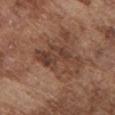Imaged during a routine full-body skin examination; the lesion was not biopsied and no histopathology is available. Located on the chest. Imaged with white-light lighting. A 15 mm close-up tile from a total-body photography series done for melanoma screening. The recorded lesion diameter is about 6 mm. Automated tile analysis of the lesion measured an area of roughly 12 mm², a shape eccentricity near 0.85, and a shape-asymmetry score of about 0.5 (0 = symmetric). The software also gave border irregularity of about 6.5 on a 0–10 scale, a within-lesion color-variation index near 3.5/10, and a peripheral color-asymmetry measure near 1. The analysis additionally found a lesion-detection confidence of about 100/100. A male subject, roughly 75 years of age.